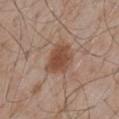Assessment: This lesion was catalogued during total-body skin photography and was not selected for biopsy.